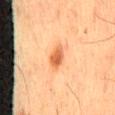This lesion was catalogued during total-body skin photography and was not selected for biopsy.
From the mid back.
The tile uses cross-polarized illumination.
Longest diameter approximately 3 mm.
A male subject, approximately 60 years of age.
Automated tile analysis of the lesion measured border irregularity of about 1.5 on a 0–10 scale, internal color variation of about 3 on a 0–10 scale, and radial color variation of about 1. It also reported a classifier nevus-likeness of about 95/100 and a lesion-detection confidence of about 100/100.
A 15 mm crop from a total-body photograph taken for skin-cancer surveillance.